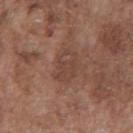Findings:
- workup · total-body-photography surveillance lesion; no biopsy
- subject · male, approximately 75 years of age
- illumination · white-light illumination
- imaging modality · ~15 mm tile from a whole-body skin photo
- lesion size · ~4 mm (longest diameter)
- location · the chest